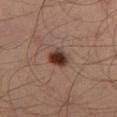Impression: No biopsy was performed on this lesion — it was imaged during a full skin examination and was not determined to be concerning. Image and clinical context: Captured under cross-polarized illumination. On the left lower leg. Automated image analysis of the tile measured an average lesion color of about L≈31 a*≈19 b*≈24 (CIELAB), about 14 CIELAB-L* units darker than the surrounding skin, and a normalized border contrast of about 13. And it measured a classifier nevus-likeness of about 100/100 and a lesion-detection confidence of about 100/100. About 2.5 mm across. A male subject, aged approximately 35. A close-up tile cropped from a whole-body skin photograph, about 15 mm across.